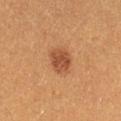Background:
A close-up tile cropped from a whole-body skin photograph, about 15 mm across. A female patient, aged approximately 40. The lesion is on the right thigh.